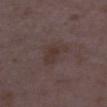No biopsy was performed on this lesion — it was imaged during a full skin examination and was not determined to be concerning.
Automated tile analysis of the lesion measured a footprint of about 6 mm² and a shape eccentricity near 0.8. The software also gave a lesion-detection confidence of about 100/100.
A 15 mm close-up extracted from a 3D total-body photography capture.
The tile uses white-light illumination.
The patient is a female roughly 35 years of age.
From the right lower leg.
Approximately 3.5 mm at its widest.